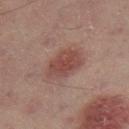Image and clinical context: Cropped from a total-body skin-imaging series; the visible field is about 15 mm. A male patient, approximately 50 years of age. Imaged with cross-polarized lighting. On the right thigh. Longest diameter approximately 5 mm.